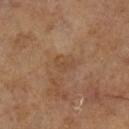Q: Is there a histopathology result?
A: catalogued during a skin exam; not biopsied
Q: What is the lesion's diameter?
A: about 2.5 mm
Q: What is the imaging modality?
A: 15 mm crop, total-body photography
Q: Who is the patient?
A: male, approximately 65 years of age
Q: Illumination type?
A: cross-polarized
Q: Automated lesion metrics?
A: a shape eccentricity near 0.75 and two-axis asymmetry of about 0.35; roughly 5 lightness units darker than nearby skin and a normalized lesion–skin contrast near 4.5; border irregularity of about 3.5 on a 0–10 scale and internal color variation of about 1 on a 0–10 scale